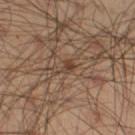Impression:
No biopsy was performed on this lesion — it was imaged during a full skin examination and was not determined to be concerning.
Clinical summary:
The lesion's longest dimension is about 2.5 mm. A 15 mm close-up extracted from a 3D total-body photography capture. The patient is a male in their 40s. Captured under cross-polarized illumination. From the leg.